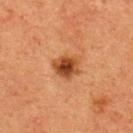subject: female, about 40 years old | lighting: cross-polarized illumination | acquisition: total-body-photography crop, ~15 mm field of view | body site: the upper back | automated metrics: an eccentricity of roughly 0.5 and a shape-asymmetry score of about 0.2 (0 = symmetric); a border-irregularity rating of about 2/10 and a within-lesion color-variation index near 5/10; an automated nevus-likeness rating near 95 out of 100 and a lesion-detection confidence of about 100/100.